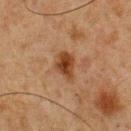| feature | finding |
|---|---|
| biopsy status | catalogued during a skin exam; not biopsied |